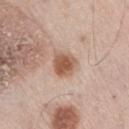diameter = ≈3 mm | location = the right thigh | subject = male, aged 73–77 | lighting = white-light | imaging modality = ~15 mm crop, total-body skin-cancer survey.